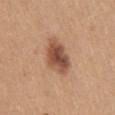Imaged during a routine full-body skin examination; the lesion was not biopsied and no histopathology is available.
A 15 mm close-up extracted from a 3D total-body photography capture.
A female patient, in their mid-30s.
The lesion is on the back.